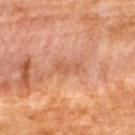{
  "biopsy_status": "not biopsied; imaged during a skin examination",
  "lesion_size": {
    "long_diameter_mm_approx": 3.0
  },
  "image": {
    "source": "total-body photography crop",
    "field_of_view_mm": 15
  },
  "site": "upper back",
  "lighting": "cross-polarized",
  "patient": {
    "sex": "female",
    "age_approx": 65
  }
}